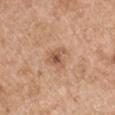A 15 mm close-up tile from a total-body photography series done for melanoma screening.
Automated image analysis of the tile measured a footprint of about 4 mm² and an eccentricity of roughly 0.5. The analysis additionally found an average lesion color of about L≈55 a*≈22 b*≈32 (CIELAB) and roughly 10 lightness units darker than nearby skin. The software also gave a border-irregularity index near 3.5/10 and a color-variation rating of about 4.5/10. And it measured an automated nevus-likeness rating near 25 out of 100 and a lesion-detection confidence of about 100/100.
Captured under white-light illumination.
A male subject aged around 55.
From the arm.